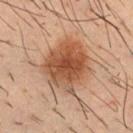Q: Was this lesion biopsied?
A: imaged on a skin check; not biopsied
Q: What kind of image is this?
A: ~15 mm crop, total-body skin-cancer survey
Q: Automated lesion metrics?
A: a lesion area of about 28 mm², an eccentricity of roughly 0.5, and a symmetry-axis asymmetry near 0.2
Q: What is the lesion's diameter?
A: ~6 mm (longest diameter)
Q: How was the tile lit?
A: cross-polarized illumination
Q: Lesion location?
A: the chest
Q: Patient demographics?
A: male, roughly 35 years of age